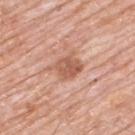A male patient, aged 78–82. Located on the upper back. The tile uses white-light illumination. Automated image analysis of the tile measured a lesion area of about 8 mm², an outline eccentricity of about 0.6 (0 = round, 1 = elongated), and two-axis asymmetry of about 0.3. A roughly 15 mm field-of-view crop from a total-body skin photograph. The recorded lesion diameter is about 4 mm.